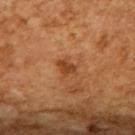| key | value |
|---|---|
| notes | total-body-photography surveillance lesion; no biopsy |
| lighting | cross-polarized |
| subject | male, roughly 65 years of age |
| lesion diameter | ≈3 mm |
| image | ~15 mm crop, total-body skin-cancer survey |
| automated metrics | a footprint of about 4 mm², an eccentricity of roughly 0.7, and a shape-asymmetry score of about 0.3 (0 = symmetric) |